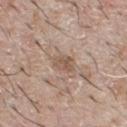Cropped from a whole-body photographic skin survey; the tile spans about 15 mm. The lesion is located on the chest. A male patient, in their mid-60s. Captured under white-light illumination.